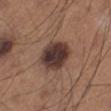notes: imaged on a skin check; not biopsied.